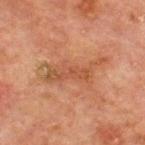biopsy status: no biopsy performed (imaged during a skin exam)
automated metrics: an area of roughly 13 mm², a shape eccentricity near 0.9, and two-axis asymmetry of about 0.45; an automated nevus-likeness rating near 0 out of 100 and lesion-presence confidence of about 100/100
image source: 15 mm crop, total-body photography
anatomic site: the front of the torso
subject: male, aged around 65
lighting: cross-polarized
diameter: about 7 mm